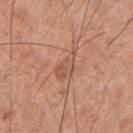This lesion was catalogued during total-body skin photography and was not selected for biopsy.
Longest diameter approximately 4 mm.
A male patient in their mid- to late 50s.
Captured under white-light illumination.
Automated image analysis of the tile measured an area of roughly 5.5 mm², a shape eccentricity near 0.85, and two-axis asymmetry of about 0.35. And it measured a border-irregularity index near 3.5/10, a within-lesion color-variation index near 2.5/10, and peripheral color asymmetry of about 1. The software also gave a classifier nevus-likeness of about 0/100 and lesion-presence confidence of about 100/100.
On the chest.
A 15 mm close-up tile from a total-body photography series done for melanoma screening.